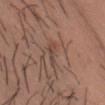Impression:
Imaged during a routine full-body skin examination; the lesion was not biopsied and no histopathology is available.
Context:
Located on the chest. A lesion tile, about 15 mm wide, cut from a 3D total-body photograph. The tile uses white-light illumination. About 3.5 mm across. An algorithmic analysis of the crop reported a footprint of about 3.5 mm², a shape eccentricity near 0.9, and two-axis asymmetry of about 0.35. And it measured a mean CIELAB color near L≈45 a*≈18 b*≈25, roughly 7 lightness units darker than nearby skin, and a lesion-to-skin contrast of about 5.5 (normalized; higher = more distinct). It also reported internal color variation of about 1.5 on a 0–10 scale and peripheral color asymmetry of about 0.5. It also reported an automated nevus-likeness rating near 0 out of 100. A male patient, about 30 years old.